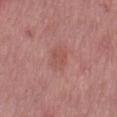{"biopsy_status": "not biopsied; imaged during a skin examination"}